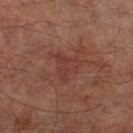Clinical impression:
Captured during whole-body skin photography for melanoma surveillance; the lesion was not biopsied.
Context:
The subject is a male aged around 65. A close-up tile cropped from a whole-body skin photograph, about 15 mm across. The lesion-visualizer software estimated an outline eccentricity of about 0.45 (0 = round, 1 = elongated) and two-axis asymmetry of about 0.55. The analysis additionally found an automated nevus-likeness rating near 0 out of 100 and a detector confidence of about 100 out of 100 that the crop contains a lesion. Located on the right lower leg. About 3.5 mm across.